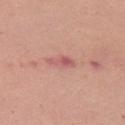Clinical impression:
Imaged during a routine full-body skin examination; the lesion was not biopsied and no histopathology is available.
Clinical summary:
This image is a 15 mm lesion crop taken from a total-body photograph. Captured under white-light illumination. From the right upper arm. Measured at roughly 3 mm in maximum diameter. A female patient, in their mid- to late 30s.